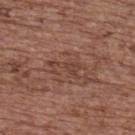| key | value |
|---|---|
| subject | female, about 65 years old |
| lesion diameter | ~4 mm (longest diameter) |
| illumination | white-light illumination |
| imaging modality | total-body-photography crop, ~15 mm field of view |
| anatomic site | the back |
| automated lesion analysis | an area of roughly 4 mm², a shape eccentricity near 0.9, and two-axis asymmetry of about 0.65; a border-irregularity rating of about 7.5/10 and a peripheral color-asymmetry measure near 0; a classifier nevus-likeness of about 0/100 and a detector confidence of about 90 out of 100 that the crop contains a lesion |